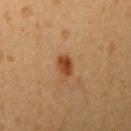No biopsy was performed on this lesion — it was imaged during a full skin examination and was not determined to be concerning.
From the right upper arm.
This is a cross-polarized tile.
A 15 mm close-up extracted from a 3D total-body photography capture.
A female subject, aged 18–22.
The recorded lesion diameter is about 2.5 mm.